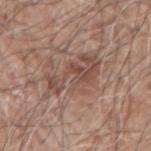follow-up: catalogued during a skin exam; not biopsied | body site: the right upper arm | subject: male, approximately 60 years of age | image: 15 mm crop, total-body photography.